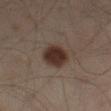Impression: Recorded during total-body skin imaging; not selected for excision or biopsy. Background: A 15 mm close-up tile from a total-body photography series done for melanoma screening. On the left thigh. A male patient, aged 58–62. Automated tile analysis of the lesion measured a mean CIELAB color near L≈28 a*≈14 b*≈20, about 12 CIELAB-L* units darker than the surrounding skin, and a normalized border contrast of about 11.5. And it measured a border-irregularity index near 1/10, a color-variation rating of about 2.5/10, and peripheral color asymmetry of about 1. Measured at roughly 4 mm in maximum diameter.